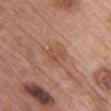| key | value |
|---|---|
| workup | no biopsy performed (imaged during a skin exam) |
| TBP lesion metrics | a footprint of about 4 mm², an eccentricity of roughly 0.65, and a shape-asymmetry score of about 0.3 (0 = symmetric) |
| subject | female, about 60 years old |
| site | the chest |
| acquisition | 15 mm crop, total-body photography |
| lighting | white-light |
| lesion diameter | ~2.5 mm (longest diameter) |